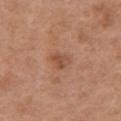biopsy status: catalogued during a skin exam; not biopsied | location: the chest | automated lesion analysis: a footprint of about 4.5 mm² and an outline eccentricity of about 0.7 (0 = round, 1 = elongated); a mean CIELAB color near L≈51 a*≈22 b*≈32 and a lesion–skin lightness drop of about 8 | image: 15 mm crop, total-body photography | lighting: white-light illumination | patient: female, approximately 65 years of age | lesion diameter: ~3 mm (longest diameter).